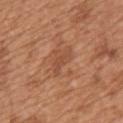Q: Was a biopsy performed?
A: catalogued during a skin exam; not biopsied
Q: Where on the body is the lesion?
A: the upper back
Q: Automated lesion metrics?
A: a lesion area of about 4 mm², an eccentricity of roughly 0.9, and a shape-asymmetry score of about 0.45 (0 = symmetric); border irregularity of about 5 on a 0–10 scale and internal color variation of about 0.5 on a 0–10 scale; a classifier nevus-likeness of about 0/100 and lesion-presence confidence of about 100/100
Q: Illumination type?
A: white-light
Q: Lesion size?
A: ~3.5 mm (longest diameter)
Q: What kind of image is this?
A: ~15 mm tile from a whole-body skin photo
Q: Patient demographics?
A: male, in their mid- to late 60s